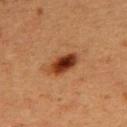Q: What lighting was used for the tile?
A: cross-polarized
Q: Lesion size?
A: about 4 mm
Q: Where on the body is the lesion?
A: the upper back
Q: Patient demographics?
A: female, roughly 40 years of age
Q: How was this image acquired?
A: total-body-photography crop, ~15 mm field of view
Q: What did automated image analysis measure?
A: roughly 14 lightness units darker than nearby skin; border irregularity of about 2.5 on a 0–10 scale, a within-lesion color-variation index near 7/10, and peripheral color asymmetry of about 2